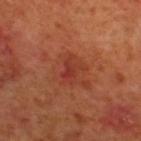| key | value |
|---|---|
| lesion size | ≈3 mm |
| image | ~15 mm tile from a whole-body skin photo |
| subject | male, about 70 years old |
| illumination | cross-polarized illumination |
| site | the upper back |